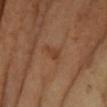Q: Was a biopsy performed?
A: total-body-photography surveillance lesion; no biopsy
Q: What kind of image is this?
A: ~15 mm crop, total-body skin-cancer survey
Q: What is the anatomic site?
A: the left forearm
Q: Illumination type?
A: cross-polarized illumination
Q: What did automated image analysis measure?
A: a footprint of about 2.5 mm², a shape eccentricity near 0.9, and a symmetry-axis asymmetry near 0.45; a mean CIELAB color near L≈41 a*≈23 b*≈33 and about 7 CIELAB-L* units darker than the surrounding skin; a nevus-likeness score of about 0/100 and a lesion-detection confidence of about 100/100
Q: Lesion size?
A: ~2.5 mm (longest diameter)
Q: Who is the patient?
A: female